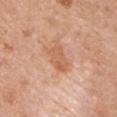image: 15 mm crop, total-body photography
tile lighting: white-light illumination
patient: female, aged approximately 50
size: about 4 mm
automated metrics: a footprint of about 7 mm² and an outline eccentricity of about 0.85 (0 = round, 1 = elongated); a lesion-detection confidence of about 100/100
location: the left upper arm